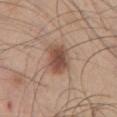Q: What is the lesion's diameter?
A: ≈3.5 mm
Q: What are the patient's age and sex?
A: male, aged approximately 45
Q: What kind of image is this?
A: ~15 mm crop, total-body skin-cancer survey
Q: Where on the body is the lesion?
A: the chest
Q: Automated lesion metrics?
A: a detector confidence of about 100 out of 100 that the crop contains a lesion